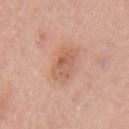Part of a total-body skin-imaging series; this lesion was reviewed on a skin check and was not flagged for biopsy.
The lesion is located on the left upper arm.
This is a white-light tile.
An algorithmic analysis of the crop reported an area of roughly 9.5 mm², an eccentricity of roughly 0.75, and two-axis asymmetry of about 0.25. The analysis additionally found a border-irregularity rating of about 2.5/10 and internal color variation of about 4.5 on a 0–10 scale.
A 15 mm close-up tile from a total-body photography series done for melanoma screening.
A female patient about 40 years old.
The lesion's longest dimension is about 4 mm.